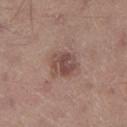The lesion was photographed on a routine skin check and not biopsied; there is no pathology result. From the right lower leg. Approximately 3 mm at its widest. Cropped from a total-body skin-imaging series; the visible field is about 15 mm. A male patient approximately 55 years of age. This is a white-light tile.